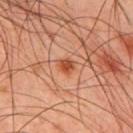Context: The lesion's longest dimension is about 2 mm. A region of skin cropped from a whole-body photographic capture, roughly 15 mm wide. On the back. The patient is a male aged around 45. Captured under cross-polarized illumination.